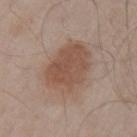Q: Patient demographics?
A: male, roughly 55 years of age
Q: Illumination type?
A: white-light
Q: What is the imaging modality?
A: ~15 mm tile from a whole-body skin photo
Q: Lesion location?
A: the left upper arm
Q: What is the lesion's diameter?
A: ~6.5 mm (longest diameter)